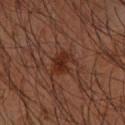The lesion is located on the arm.
A 15 mm crop from a total-body photograph taken for skin-cancer surveillance.
A male subject, aged around 60.